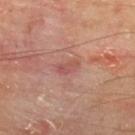• follow-up — no biopsy performed (imaged during a skin exam)
• lesion size — about 3 mm
• image source — ~15 mm crop, total-body skin-cancer survey
• patient — male, aged 63 to 67
• body site — the upper back
• illumination — cross-polarized